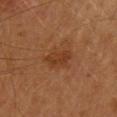Q: Is there a histopathology result?
A: total-body-photography surveillance lesion; no biopsy
Q: What is the anatomic site?
A: the upper back
Q: Automated lesion metrics?
A: a mean CIELAB color near L≈32 a*≈21 b*≈31, roughly 6 lightness units darker than nearby skin, and a normalized lesion–skin contrast near 6.5; a border-irregularity index near 4/10 and a color-variation rating of about 3.5/10
Q: Lesion size?
A: about 3.5 mm
Q: How was the tile lit?
A: cross-polarized
Q: Patient demographics?
A: female, aged 58 to 62
Q: What kind of image is this?
A: 15 mm crop, total-body photography